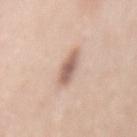follow-up: no biopsy performed (imaged during a skin exam) | automated lesion analysis: a lesion area of about 5.5 mm², an eccentricity of roughly 0.9, and two-axis asymmetry of about 0.25 | anatomic site: the mid back | acquisition: ~15 mm tile from a whole-body skin photo | patient: female, aged around 50 | lesion size: ~4 mm (longest diameter) | illumination: white-light illumination.